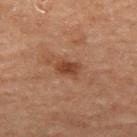Impression:
The lesion was tiled from a total-body skin photograph and was not biopsied.
Acquisition and patient details:
A lesion tile, about 15 mm wide, cut from a 3D total-body photograph. The total-body-photography lesion software estimated an automated nevus-likeness rating near 85 out of 100 and a detector confidence of about 100 out of 100 that the crop contains a lesion. Captured under cross-polarized illumination. Longest diameter approximately 2.5 mm. The subject is a male aged approximately 70. On the left thigh.